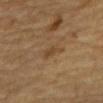Background: A male patient approximately 85 years of age or older. A 15 mm close-up extracted from a 3D total-body photography capture. The total-body-photography lesion software estimated a lesion color around L≈37 a*≈14 b*≈29 in CIELAB and a lesion–skin lightness drop of about 6. The software also gave a within-lesion color-variation index near 0.5/10. The software also gave a classifier nevus-likeness of about 0/100 and lesion-presence confidence of about 100/100. Captured under cross-polarized illumination. The lesion is located on the mid back. Approximately 2.5 mm at its widest.